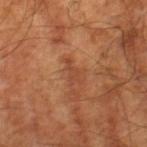Q: Was a biopsy performed?
A: catalogued during a skin exam; not biopsied
Q: What is the lesion's diameter?
A: ≈3 mm
Q: Automated lesion metrics?
A: a classifier nevus-likeness of about 0/100 and a lesion-detection confidence of about 100/100
Q: How was this image acquired?
A: ~15 mm tile from a whole-body skin photo
Q: What are the patient's age and sex?
A: male, approximately 60 years of age
Q: What is the anatomic site?
A: the right thigh
Q: Illumination type?
A: cross-polarized illumination